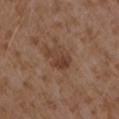Imaged during a routine full-body skin examination; the lesion was not biopsied and no histopathology is available. Cropped from a whole-body photographic skin survey; the tile spans about 15 mm. The subject is a female aged around 35. The tile uses white-light illumination. Located on the arm.